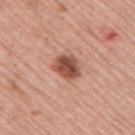Clinical summary:
The subject is a male aged approximately 55. This is a white-light tile. Cropped from a whole-body photographic skin survey; the tile spans about 15 mm. Measured at roughly 3.5 mm in maximum diameter. The lesion is on the right upper arm.